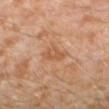workup: imaged on a skin check; not biopsied | image-analysis metrics: a lesion color around L≈54 a*≈22 b*≈35 in CIELAB and a lesion–skin lightness drop of about 7 | image source: ~15 mm crop, total-body skin-cancer survey | site: the left lower leg | lesion diameter: ~3 mm (longest diameter) | tile lighting: cross-polarized | subject: male, aged 28–32.